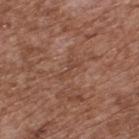{"biopsy_status": "not biopsied; imaged during a skin examination", "lesion_size": {"long_diameter_mm_approx": 3.0}, "lighting": "white-light", "patient": {"sex": "male", "age_approx": 75}, "site": "upper back", "image": {"source": "total-body photography crop", "field_of_view_mm": 15}}